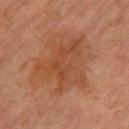The lesion was tiled from a total-body skin photograph and was not biopsied. A female patient in their mid- to late 60s. A 15 mm close-up extracted from a 3D total-body photography capture. From the leg.